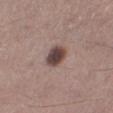Notes:
– biopsy status: imaged on a skin check; not biopsied
– subject: male, aged around 45
– size: ≈3 mm
– image source: total-body-photography crop, ~15 mm field of view
– anatomic site: the left lower leg
– automated lesion analysis: a footprint of about 6.5 mm², an eccentricity of roughly 0.6, and a shape-asymmetry score of about 0.1 (0 = symmetric); border irregularity of about 1 on a 0–10 scale and a peripheral color-asymmetry measure near 1; an automated nevus-likeness rating near 80 out of 100